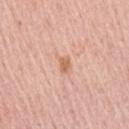Imaged during a routine full-body skin examination; the lesion was not biopsied and no histopathology is available.
This is a white-light tile.
From the right upper arm.
A lesion tile, about 15 mm wide, cut from a 3D total-body photograph.
An algorithmic analysis of the crop reported a lesion area of about 5 mm², an eccentricity of roughly 0.8, and a shape-asymmetry score of about 0.35 (0 = symmetric). The software also gave a within-lesion color-variation index near 3.5/10 and a peripheral color-asymmetry measure near 1.
Longest diameter approximately 3.5 mm.
A male patient, approximately 65 years of age.